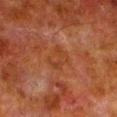Clinical impression: Captured during whole-body skin photography for melanoma surveillance; the lesion was not biopsied. Acquisition and patient details: Measured at roughly 3 mm in maximum diameter. The total-body-photography lesion software estimated an area of roughly 6.5 mm². The analysis additionally found a lesion color around L≈32 a*≈22 b*≈30 in CIELAB and roughly 4 lightness units darker than nearby skin. The analysis additionally found a detector confidence of about 100 out of 100 that the crop contains a lesion. Captured under cross-polarized illumination. The lesion is located on the left lower leg. A male patient, aged 78 to 82. A roughly 15 mm field-of-view crop from a total-body skin photograph.